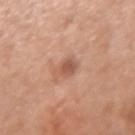<lesion>
  <biopsy_status>not biopsied; imaged during a skin examination</biopsy_status>
  <site>left forearm</site>
  <patient>
    <sex>female</sex>
    <age_approx>50</age_approx>
  </patient>
  <image>
    <source>total-body photography crop</source>
    <field_of_view_mm>15</field_of_view_mm>
  </image>
  <lighting>white-light</lighting>
  <lesion_size>
    <long_diameter_mm_approx>3.0</long_diameter_mm_approx>
  </lesion_size>
  <automated_metrics>
    <cielab_L>55</cielab_L>
    <cielab_a>23</cielab_a>
    <cielab_b>31</cielab_b>
    <vs_skin_darker_L>10.0</vs_skin_darker_L>
    <vs_skin_contrast_norm>7.0</vs_skin_contrast_norm>
    <nevus_likeness_0_100>25</nevus_likeness_0_100>
  </automated_metrics>
</lesion>